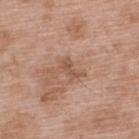Notes:
- acquisition: ~15 mm crop, total-body skin-cancer survey
- site: the upper back
- subject: male, in their 50s